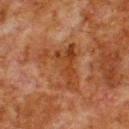Findings:
– follow-up · total-body-photography surveillance lesion; no biopsy
– acquisition · total-body-photography crop, ~15 mm field of view
– subject · male, aged around 80
– site · the upper back